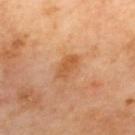Q: Where on the body is the lesion?
A: the upper back
Q: How was this image acquired?
A: ~15 mm tile from a whole-body skin photo